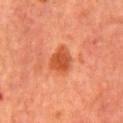<record>
  <biopsy_status>not biopsied; imaged during a skin examination</biopsy_status>
  <automated_metrics>
    <area_mm2_approx>7.5</area_mm2_approx>
    <shape_asymmetry>0.35</shape_asymmetry>
    <vs_skin_darker_L>10.0</vs_skin_darker_L>
    <vs_skin_contrast_norm>8.0</vs_skin_contrast_norm>
    <nevus_likeness_0_100>90</nevus_likeness_0_100>
    <lesion_detection_confidence_0_100>100</lesion_detection_confidence_0_100>
  </automated_metrics>
  <site>front of the torso</site>
  <image>
    <source>total-body photography crop</source>
    <field_of_view_mm>15</field_of_view_mm>
  </image>
  <patient>
    <sex>male</sex>
    <age_approx>65</age_approx>
  </patient>
  <lesion_size>
    <long_diameter_mm_approx>4.0</long_diameter_mm_approx>
  </lesion_size>
</record>